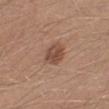<case>
  <biopsy_status>not biopsied; imaged during a skin examination</biopsy_status>
  <patient>
    <sex>male</sex>
    <age_approx>30</age_approx>
  </patient>
  <automated_metrics>
    <area_mm2_approx>6.5</area_mm2_approx>
    <eccentricity>0.45</eccentricity>
    <shape_asymmetry>0.15</shape_asymmetry>
    <color_variation_0_10>3.5</color_variation_0_10>
    <peripheral_color_asymmetry>1.0</peripheral_color_asymmetry>
    <nevus_likeness_0_100>70</nevus_likeness_0_100>
    <lesion_detection_confidence_0_100>100</lesion_detection_confidence_0_100>
  </automated_metrics>
  <lighting>white-light</lighting>
  <image>
    <source>total-body photography crop</source>
    <field_of_view_mm>15</field_of_view_mm>
  </image>
  <lesion_size>
    <long_diameter_mm_approx>3.0</long_diameter_mm_approx>
  </lesion_size>
  <site>leg</site>
</case>